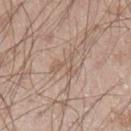Notes:
– imaging modality: ~15 mm tile from a whole-body skin photo
– anatomic site: the left lower leg
– automated metrics: a footprint of about 3.5 mm², an eccentricity of roughly 0.7, and two-axis asymmetry of about 0.75; a mean CIELAB color near L≈57 a*≈16 b*≈26 and about 6 CIELAB-L* units darker than the surrounding skin; a border-irregularity rating of about 8.5/10 and a color-variation rating of about 0/10
– patient: male, about 35 years old
– lesion size: ~3 mm (longest diameter)